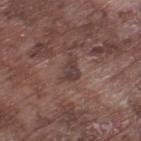The lesion was tiled from a total-body skin photograph and was not biopsied. On the leg. Cropped from a whole-body photographic skin survey; the tile spans about 15 mm. This is a white-light tile. A male patient, about 75 years old.